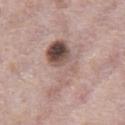notes = catalogued during a skin exam; not biopsied | lighting = white-light | acquisition = ~15 mm tile from a whole-body skin photo | subject = male, about 70 years old | image-analysis metrics = an automated nevus-likeness rating near 10 out of 100 and a lesion-detection confidence of about 100/100 | anatomic site = the chest | lesion size = ≈7.5 mm.